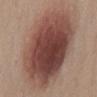Imaged during a routine full-body skin examination; the lesion was not biopsied and no histopathology is available. The lesion is on the chest. The recorded lesion diameter is about 11.5 mm. This is a white-light tile. A female subject aged around 45. This image is a 15 mm lesion crop taken from a total-body photograph.